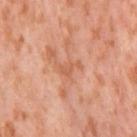Assessment: Part of a total-body skin-imaging series; this lesion was reviewed on a skin check and was not flagged for biopsy. Context: A roughly 15 mm field-of-view crop from a total-body skin photograph. Located on the right thigh. The subject is a female in their mid-50s.